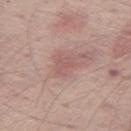workup: total-body-photography surveillance lesion; no biopsy
anatomic site: the left thigh
subject: male, roughly 65 years of age
illumination: white-light
automated metrics: a footprint of about 4 mm², an outline eccentricity of about 0.7 (0 = round, 1 = elongated), and two-axis asymmetry of about 0.35; a lesion color around L≈56 a*≈21 b*≈22 in CIELAB, about 6 CIELAB-L* units darker than the surrounding skin, and a normalized lesion–skin contrast near 4.5; an automated nevus-likeness rating near 0 out of 100 and a detector confidence of about 100 out of 100 that the crop contains a lesion
image source: total-body-photography crop, ~15 mm field of view
size: ≈2.5 mm